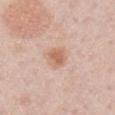A 15 mm close-up extracted from a 3D total-body photography capture.
Automated image analysis of the tile measured an area of roughly 5.5 mm² and a symmetry-axis asymmetry near 0.25. The software also gave a nevus-likeness score of about 80/100 and lesion-presence confidence of about 100/100.
Measured at roughly 2.5 mm in maximum diameter.
A male subject, about 60 years old.
The lesion is located on the right upper arm.
Captured under white-light illumination.